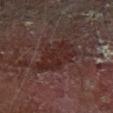{
  "biopsy_status": "not biopsied; imaged during a skin examination",
  "lesion_size": {
    "long_diameter_mm_approx": 6.0
  },
  "site": "left forearm",
  "patient": {
    "sex": "male",
    "age_approx": 70
  },
  "image": {
    "source": "total-body photography crop",
    "field_of_view_mm": 15
  }
}